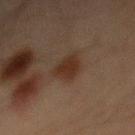Assessment: The lesion was tiled from a total-body skin photograph and was not biopsied. Clinical summary: Located on the abdomen. A lesion tile, about 15 mm wide, cut from a 3D total-body photograph. A male subject, approximately 70 years of age.